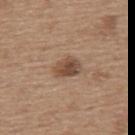follow-up = imaged on a skin check; not biopsied | patient = male, approximately 65 years of age | image source = 15 mm crop, total-body photography | location = the upper back.